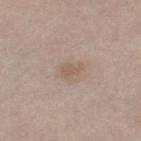notes=no biopsy performed (imaged during a skin exam); site=the left thigh; subject=male, in their mid- to late 60s; imaging modality=15 mm crop, total-body photography.